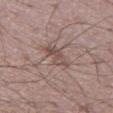notes: total-body-photography surveillance lesion; no biopsy